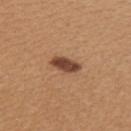This lesion was catalogued during total-body skin photography and was not selected for biopsy.
The tile uses white-light illumination.
Measured at roughly 3.5 mm in maximum diameter.
From the left upper arm.
A female patient, in their 40s.
This image is a 15 mm lesion crop taken from a total-body photograph.